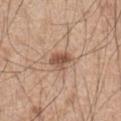illumination — white-light illumination
lesion size — about 3 mm
patient — male, aged around 70
acquisition — 15 mm crop, total-body photography
TBP lesion metrics — an area of roughly 5 mm² and a shape-asymmetry score of about 0.35 (0 = symmetric); a lesion color around L≈53 a*≈20 b*≈29 in CIELAB; a color-variation rating of about 3.5/10 and radial color variation of about 1.5; a detector confidence of about 100 out of 100 that the crop contains a lesion
location — the abdomen